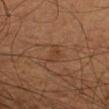This lesion was catalogued during total-body skin photography and was not selected for biopsy.
The subject is a male about 55 years old.
A 15 mm crop from a total-body photograph taken for skin-cancer surveillance.
From the arm.